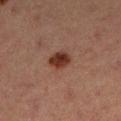Recorded during total-body skin imaging; not selected for excision or biopsy.
An algorithmic analysis of the crop reported a lesion area of about 5.5 mm², an eccentricity of roughly 0.5, and two-axis asymmetry of about 0.15. It also reported roughly 11 lightness units darker than nearby skin and a normalized lesion–skin contrast near 11.
A lesion tile, about 15 mm wide, cut from a 3D total-body photograph.
Measured at roughly 3 mm in maximum diameter.
A female patient aged approximately 40.
The tile uses cross-polarized illumination.
On the left lower leg.